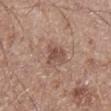biopsy status: catalogued during a skin exam; not biopsied
site: the right lower leg
acquisition: ~15 mm crop, total-body skin-cancer survey
patient: male, approximately 60 years of age
lighting: white-light